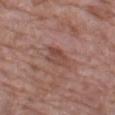The lesion was tiled from a total-body skin photograph and was not biopsied. Imaged with white-light lighting. The subject is a female roughly 70 years of age. From the left thigh. This image is a 15 mm lesion crop taken from a total-body photograph. Approximately 3.5 mm at its widest.